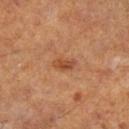The lesion was photographed on a routine skin check and not biopsied; there is no pathology result. A close-up tile cropped from a whole-body skin photograph, about 15 mm across. Longest diameter approximately 3 mm. Imaged with cross-polarized lighting. A male subject, approximately 60 years of age. Located on the left lower leg.